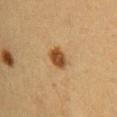This lesion was catalogued during total-body skin photography and was not selected for biopsy. A female patient aged approximately 30. The tile uses cross-polarized illumination. The recorded lesion diameter is about 3 mm. The lesion-visualizer software estimated an area of roughly 6 mm², an outline eccentricity of about 0.7 (0 = round, 1 = elongated), and a symmetry-axis asymmetry near 0.2. The analysis additionally found an average lesion color of about L≈46 a*≈19 b*≈36 (CIELAB), a lesion–skin lightness drop of about 13, and a lesion-to-skin contrast of about 10 (normalized; higher = more distinct). The software also gave a nevus-likeness score of about 100/100 and a detector confidence of about 100 out of 100 that the crop contains a lesion. A roughly 15 mm field-of-view crop from a total-body skin photograph. Located on the right upper arm.